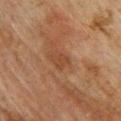* workup — catalogued during a skin exam; not biopsied
* imaging modality — ~15 mm tile from a whole-body skin photo
* body site — the upper back
* subject — male, aged approximately 75